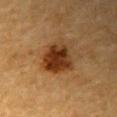patient = male, in their mid- to late 80s or older
location = the right upper arm
imaging modality = 15 mm crop, total-body photography
tile lighting = cross-polarized illumination
lesion size = ~4.5 mm (longest diameter)